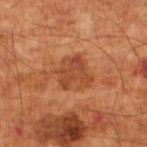workup = catalogued during a skin exam; not biopsied | anatomic site = the left lower leg | automated lesion analysis = an area of roughly 10 mm², an eccentricity of roughly 0.3, and a symmetry-axis asymmetry near 0.3; a within-lesion color-variation index near 4.5/10 and a peripheral color-asymmetry measure near 1.5; a classifier nevus-likeness of about 35/100 and a lesion-detection confidence of about 100/100 | patient = male, aged approximately 65 | acquisition = ~15 mm crop, total-body skin-cancer survey | size = ≈3.5 mm | lighting = cross-polarized.